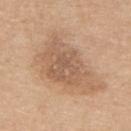<tbp_lesion>
  <biopsy_status>not biopsied; imaged during a skin examination</biopsy_status>
</tbp_lesion>